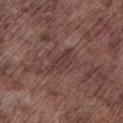Imaged during a routine full-body skin examination; the lesion was not biopsied and no histopathology is available. Located on the left lower leg. A close-up tile cropped from a whole-body skin photograph, about 15 mm across. A male subject in their mid-70s. Captured under white-light illumination. About 4 mm across.